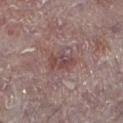Q: Is there a histopathology result?
A: total-body-photography surveillance lesion; no biopsy
Q: How large is the lesion?
A: ~3.5 mm (longest diameter)
Q: Patient demographics?
A: male, aged 73 to 77
Q: What is the anatomic site?
A: the left lower leg
Q: What did automated image analysis measure?
A: a footprint of about 5.5 mm² and a symmetry-axis asymmetry near 0.3
Q: What lighting was used for the tile?
A: white-light illumination
Q: What kind of image is this?
A: 15 mm crop, total-body photography